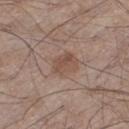Clinical impression:
No biopsy was performed on this lesion — it was imaged during a full skin examination and was not determined to be concerning.
Clinical summary:
This image is a 15 mm lesion crop taken from a total-body photograph. Imaged with white-light lighting. The lesion is located on the leg. Measured at roughly 3 mm in maximum diameter. The lesion-visualizer software estimated an area of roughly 6.5 mm² and a symmetry-axis asymmetry near 0.2. And it measured a mean CIELAB color near L≈50 a*≈17 b*≈25 and roughly 8 lightness units darker than nearby skin. And it measured border irregularity of about 2 on a 0–10 scale, a within-lesion color-variation index near 3.5/10, and peripheral color asymmetry of about 1.5. A male patient, approximately 55 years of age.